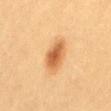– patient: female, roughly 20 years of age
– lighting: cross-polarized illumination
– diameter: ≈4.5 mm
– image: ~15 mm tile from a whole-body skin photo
– anatomic site: the mid back